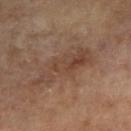The lesion was photographed on a routine skin check and not biopsied; there is no pathology result. This is a cross-polarized tile. A 15 mm crop from a total-body photograph taken for skin-cancer surveillance. On the arm. A female patient, aged 63 to 67. Measured at roughly 7.5 mm in maximum diameter.